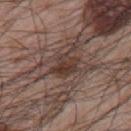{"biopsy_status": "not biopsied; imaged during a skin examination", "lesion_size": {"long_diameter_mm_approx": 3.5}, "lighting": "white-light", "site": "arm", "patient": {"sex": "male", "age_approx": 65}, "image": {"source": "total-body photography crop", "field_of_view_mm": 15}}